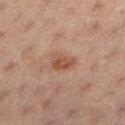Part of a total-body skin-imaging series; this lesion was reviewed on a skin check and was not flagged for biopsy. The lesion's longest dimension is about 3.5 mm. A lesion tile, about 15 mm wide, cut from a 3D total-body photograph. Imaged with cross-polarized lighting. The lesion is located on the left lower leg. A female subject in their 20s. Automated image analysis of the tile measured an average lesion color of about L≈44 a*≈19 b*≈26 (CIELAB), a lesion–skin lightness drop of about 8, and a normalized border contrast of about 7. And it measured border irregularity of about 3 on a 0–10 scale, a color-variation rating of about 3/10, and peripheral color asymmetry of about 1.